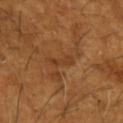Impression: Imaged during a routine full-body skin examination; the lesion was not biopsied and no histopathology is available. Context: A roughly 15 mm field-of-view crop from a total-body skin photograph. On the arm. Captured under cross-polarized illumination. The subject is a male aged 63–67. Approximately 2.5 mm at its widest. An algorithmic analysis of the crop reported an area of roughly 3 mm², an eccentricity of roughly 0.9, and two-axis asymmetry of about 0.4.